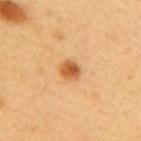Findings:
* automated lesion analysis — about 14 CIELAB-L* units darker than the surrounding skin and a lesion-to-skin contrast of about 9 (normalized; higher = more distinct); border irregularity of about 1 on a 0–10 scale, a color-variation rating of about 4.5/10, and a peripheral color-asymmetry measure near 1.5; a classifier nevus-likeness of about 100/100 and a lesion-detection confidence of about 100/100
* patient — female, about 55 years old
* location — the back
* lighting — cross-polarized
* imaging modality — ~15 mm crop, total-body skin-cancer survey